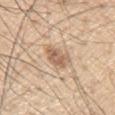  biopsy_status: not biopsied; imaged during a skin examination
  image:
    source: total-body photography crop
    field_of_view_mm: 15
  patient:
    sex: male
    age_approx: 55
  lesion_size:
    long_diameter_mm_approx: 3.0
  site: left upper arm
  lighting: white-light
  automated_metrics:
    area_mm2_approx: 6.5
    eccentricity: 0.5
    cielab_L: 61
    cielab_a: 17
    cielab_b: 31
    vs_skin_darker_L: 11.0
    vs_skin_contrast_norm: 7.0
    border_irregularity_0_10: 2.5
    peripheral_color_asymmetry: 1.5
    nevus_likeness_0_100: 45
    lesion_detection_confidence_0_100: 100